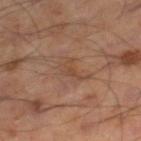Notes:
- follow-up — catalogued during a skin exam; not biopsied
- anatomic site — the right lower leg
- acquisition — total-body-photography crop, ~15 mm field of view
- lesion size — about 3 mm
- patient — male, about 55 years old
- lighting — cross-polarized